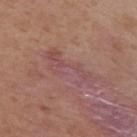The lesion was photographed on a routine skin check and not biopsied; there is no pathology result.
From the upper back.
An algorithmic analysis of the crop reported a footprint of about 10 mm² and a symmetry-axis asymmetry near 0.55. And it measured a lesion color around L≈49 a*≈23 b*≈20 in CIELAB and a lesion–skin lightness drop of about 6. The software also gave a classifier nevus-likeness of about 0/100 and lesion-presence confidence of about 60/100.
A region of skin cropped from a whole-body photographic capture, roughly 15 mm wide.
The lesion's longest dimension is about 6.5 mm.
This is a white-light tile.
A female subject about 40 years old.